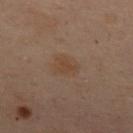{
  "biopsy_status": "not biopsied; imaged during a skin examination",
  "patient": {
    "sex": "female",
    "age_approx": 55
  },
  "lighting": "cross-polarized",
  "lesion_size": {
    "long_diameter_mm_approx": 2.5
  },
  "site": "upper back",
  "image": {
    "source": "total-body photography crop",
    "field_of_view_mm": 15
  },
  "automated_metrics": {
    "area_mm2_approx": 3.0,
    "eccentricity": 0.8,
    "shape_asymmetry": 0.4,
    "cielab_L": 34,
    "cielab_a": 14,
    "cielab_b": 25,
    "vs_skin_contrast_norm": 6.0
  }
}